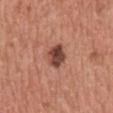Q: Was a biopsy performed?
A: catalogued during a skin exam; not biopsied
Q: What kind of image is this?
A: ~15 mm crop, total-body skin-cancer survey
Q: How large is the lesion?
A: about 3 mm
Q: Illumination type?
A: white-light illumination
Q: Patient demographics?
A: male, aged 43–47
Q: What is the anatomic site?
A: the chest